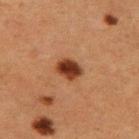| field | value |
|---|---|
| biopsy status | no biopsy performed (imaged during a skin exam) |
| acquisition | ~15 mm crop, total-body skin-cancer survey |
| size | ≈3 mm |
| subject | female, approximately 50 years of age |
| body site | the left thigh |
| image-analysis metrics | a lesion area of about 6 mm² and an eccentricity of roughly 0.65; a lesion color around L≈32 a*≈22 b*≈29 in CIELAB and roughly 14 lightness units darker than nearby skin; a border-irregularity rating of about 1.5/10, a color-variation rating of about 4/10, and peripheral color asymmetry of about 1.5; a classifier nevus-likeness of about 100/100 and a detector confidence of about 100 out of 100 that the crop contains a lesion |